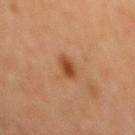biopsy status: catalogued during a skin exam; not biopsied | anatomic site: the back | image: ~15 mm crop, total-body skin-cancer survey | automated metrics: internal color variation of about 2.5 on a 0–10 scale and peripheral color asymmetry of about 0.5; an automated nevus-likeness rating near 95 out of 100 and a detector confidence of about 100 out of 100 that the crop contains a lesion | subject: male, in their mid-60s | diameter: ~3 mm (longest diameter).